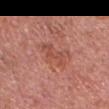Q: Was this lesion biopsied?
A: imaged on a skin check; not biopsied
Q: What lighting was used for the tile?
A: white-light illumination
Q: What are the patient's age and sex?
A: male, aged around 70
Q: What is the imaging modality?
A: ~15 mm tile from a whole-body skin photo
Q: What is the anatomic site?
A: the head or neck
Q: Lesion size?
A: about 4.5 mm